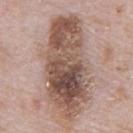notes=catalogued during a skin exam; not biopsied
site=the abdomen
diameter=about 12.5 mm
acquisition=15 mm crop, total-body photography
patient=male, in their 70s